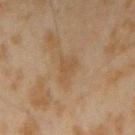<case>
  <biopsy_status>not biopsied; imaged during a skin examination</biopsy_status>
  <patient>
    <sex>male</sex>
    <age_approx>45</age_approx>
  </patient>
  <site>left forearm</site>
  <image>
    <source>total-body photography crop</source>
    <field_of_view_mm>15</field_of_view_mm>
  </image>
</case>